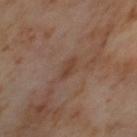{"biopsy_status": "not biopsied; imaged during a skin examination", "image": {"source": "total-body photography crop", "field_of_view_mm": 15}, "patient": {"sex": "female", "age_approx": 55}, "site": "left thigh"}